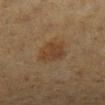Background:
This is a cross-polarized tile. Located on the left lower leg. This image is a 15 mm lesion crop taken from a total-body photograph. Measured at roughly 3.5 mm in maximum diameter. A female patient, in their mid-50s.